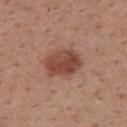Part of a total-body skin-imaging series; this lesion was reviewed on a skin check and was not flagged for biopsy. Imaged with white-light lighting. The patient is a male about 55 years old. From the mid back. A lesion tile, about 15 mm wide, cut from a 3D total-body photograph.